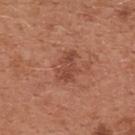diameter: about 4 mm
tile lighting: white-light illumination
image-analysis metrics: an average lesion color of about L≈46 a*≈25 b*≈30 (CIELAB), roughly 8 lightness units darker than nearby skin, and a lesion-to-skin contrast of about 6.5 (normalized; higher = more distinct); a color-variation rating of about 3/10 and a peripheral color-asymmetry measure near 1; a detector confidence of about 100 out of 100 that the crop contains a lesion
acquisition: ~15 mm tile from a whole-body skin photo
body site: the chest
subject: female, in their mid-30s